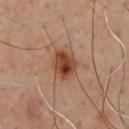workup = no biopsy performed (imaged during a skin exam)
lighting = cross-polarized
lesion diameter = ≈3.5 mm
patient = male, aged 53 to 57
site = the chest
imaging modality = ~15 mm crop, total-body skin-cancer survey